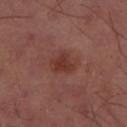Assessment: Imaged during a routine full-body skin examination; the lesion was not biopsied and no histopathology is available. Context: A region of skin cropped from a whole-body photographic capture, roughly 15 mm wide. Captured under cross-polarized illumination. The lesion's longest dimension is about 3.5 mm. The lesion is located on the left thigh.